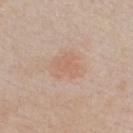Recorded during total-body skin imaging; not selected for excision or biopsy.
This image is a 15 mm lesion crop taken from a total-body photograph.
From the chest.
Imaged with white-light lighting.
A male patient, about 45 years old.